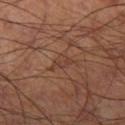Assessment: Captured during whole-body skin photography for melanoma surveillance; the lesion was not biopsied. Acquisition and patient details: A male patient, roughly 60 years of age. The lesion is on the left thigh. Cropped from a total-body skin-imaging series; the visible field is about 15 mm. The tile uses cross-polarized illumination.